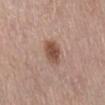subject: female, in their mid- to late 60s | location: the abdomen | image: total-body-photography crop, ~15 mm field of view | automated metrics: a lesion area of about 6.5 mm², an outline eccentricity of about 0.75 (0 = round, 1 = elongated), and two-axis asymmetry of about 0.2; a lesion color around L≈50 a*≈20 b*≈27 in CIELAB, roughly 12 lightness units darker than nearby skin, and a normalized border contrast of about 9; border irregularity of about 2 on a 0–10 scale, a color-variation rating of about 3/10, and radial color variation of about 1; an automated nevus-likeness rating near 95 out of 100 and a lesion-detection confidence of about 100/100 | tile lighting: white-light illumination | size: about 3.5 mm.